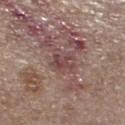follow-up=imaged on a skin check; not biopsied | image source=total-body-photography crop, ~15 mm field of view | anatomic site=the leg | patient=female, in their mid- to late 60s | diameter=~3 mm (longest diameter).